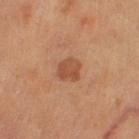follow-up: total-body-photography surveillance lesion; no biopsy
patient: aged approximately 60
anatomic site: the right thigh
diameter: ≈2.5 mm
tile lighting: cross-polarized illumination
image source: ~15 mm tile from a whole-body skin photo
TBP lesion metrics: a footprint of about 5.5 mm², an eccentricity of roughly 0.45, and a shape-asymmetry score of about 0.2 (0 = symmetric); a border-irregularity index near 2/10, a within-lesion color-variation index near 3/10, and radial color variation of about 1; a nevus-likeness score of about 40/100 and a lesion-detection confidence of about 100/100